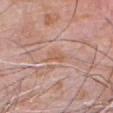lesion_size:
  long_diameter_mm_approx: 3.0
image:
  source: total-body photography crop
  field_of_view_mm: 15
automated_metrics:
  area_mm2_approx: 3.5
  eccentricity: 0.85
  shape_asymmetry: 0.35
  vs_skin_darker_L: 6.0
  vs_skin_contrast_norm: 6.5
  border_irregularity_0_10: 3.5
  peripheral_color_asymmetry: 0.5
patient:
  sex: male
  age_approx: 80
site: head or neck
lighting: white-light